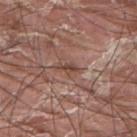Impression: No biopsy was performed on this lesion — it was imaged during a full skin examination and was not determined to be concerning. Clinical summary: The patient is a male aged approximately 40. This image is a 15 mm lesion crop taken from a total-body photograph. Longest diameter approximately 2.5 mm. On the upper back. The tile uses white-light illumination.